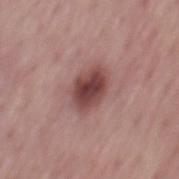Assessment: The lesion was photographed on a routine skin check and not biopsied; there is no pathology result.